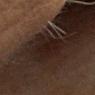Q: Is there a histopathology result?
A: no biopsy performed (imaged during a skin exam)
Q: What is the imaging modality?
A: 15 mm crop, total-body photography
Q: Lesion size?
A: ~5 mm (longest diameter)
Q: Where on the body is the lesion?
A: the chest
Q: What lighting was used for the tile?
A: cross-polarized
Q: What are the patient's age and sex?
A: female, in their 80s